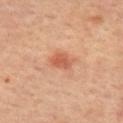A female patient, approximately 45 years of age.
The lesion's longest dimension is about 2.5 mm.
A close-up tile cropped from a whole-body skin photograph, about 15 mm across.
Automated image analysis of the tile measured a lesion area of about 4.5 mm², an eccentricity of roughly 0.65, and a symmetry-axis asymmetry near 0.2. The software also gave a border-irregularity index near 2/10, internal color variation of about 2.5 on a 0–10 scale, and radial color variation of about 1. The software also gave a nevus-likeness score of about 75/100 and lesion-presence confidence of about 100/100.
On the mid back.